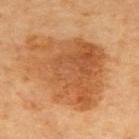biopsy status: imaged on a skin check; not biopsied
image: ~15 mm tile from a whole-body skin photo
patient: roughly 70 years of age
anatomic site: the upper back
automated lesion analysis: an area of roughly 50 mm² and a shape-asymmetry score of about 0.3 (0 = symmetric); roughly 11 lightness units darker than nearby skin and a normalized lesion–skin contrast near 7.5; a within-lesion color-variation index near 5.5/10 and peripheral color asymmetry of about 2
illumination: cross-polarized illumination
size: ≈10 mm